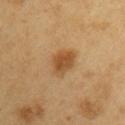• biopsy status: total-body-photography surveillance lesion; no biopsy
• TBP lesion metrics: a lesion area of about 8 mm², an outline eccentricity of about 0.7 (0 = round, 1 = elongated), and a symmetry-axis asymmetry near 0.25
• image source: total-body-photography crop, ~15 mm field of view
• anatomic site: the arm
• patient: male, aged 58–62